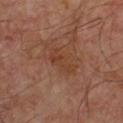Clinical impression:
The lesion was tiled from a total-body skin photograph and was not biopsied.
Context:
The subject is a male in their mid- to late 50s. The lesion is located on the chest. Captured under cross-polarized illumination. A lesion tile, about 15 mm wide, cut from a 3D total-body photograph.